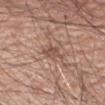workup=no biopsy performed (imaged during a skin exam) | automated lesion analysis=an eccentricity of roughly 0.8 and a symmetry-axis asymmetry near 0.35; border irregularity of about 4 on a 0–10 scale, a within-lesion color-variation index near 2/10, and a peripheral color-asymmetry measure near 1; a classifier nevus-likeness of about 0/100 | tile lighting=white-light | lesion diameter=~3 mm (longest diameter) | body site=the right upper arm | subject=male, aged around 60 | image=~15 mm crop, total-body skin-cancer survey.